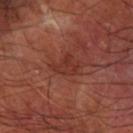Clinical impression: This lesion was catalogued during total-body skin photography and was not selected for biopsy. Context: The lesion's longest dimension is about 3 mm. From the right forearm. A 15 mm close-up extracted from a 3D total-body photography capture. Captured under cross-polarized illumination. The subject is a male aged approximately 65.